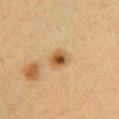{
  "biopsy_status": "not biopsied; imaged during a skin examination",
  "image": {
    "source": "total-body photography crop",
    "field_of_view_mm": 15
  },
  "lighting": "cross-polarized",
  "site": "right upper arm",
  "patient": {
    "sex": "female",
    "age_approx": 40
  },
  "lesion_size": {
    "long_diameter_mm_approx": 2.5
  }
}